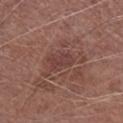Context: This image is a 15 mm lesion crop taken from a total-body photograph. The patient is a male approximately 75 years of age. On the right lower leg.